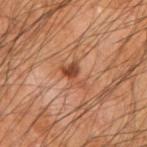Part of a total-body skin-imaging series; this lesion was reviewed on a skin check and was not flagged for biopsy.
A male patient, aged approximately 65.
From the right upper arm.
This is a cross-polarized tile.
A 15 mm close-up extracted from a 3D total-body photography capture.
The lesion's longest dimension is about 3.5 mm.